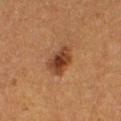Captured during whole-body skin photography for melanoma surveillance; the lesion was not biopsied. Captured under cross-polarized illumination. This image is a 15 mm lesion crop taken from a total-body photograph. The lesion is on the leg. Automated tile analysis of the lesion measured a lesion area of about 8 mm² and an outline eccentricity of about 0.65 (0 = round, 1 = elongated). It also reported an average lesion color of about L≈33 a*≈20 b*≈28 (CIELAB) and a normalized border contrast of about 9.5. It also reported border irregularity of about 2.5 on a 0–10 scale, a color-variation rating of about 4.5/10, and radial color variation of about 1. And it measured a detector confidence of about 100 out of 100 that the crop contains a lesion. A female patient, aged around 40. Approximately 3.5 mm at its widest.